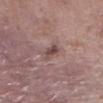workup: total-body-photography surveillance lesion; no biopsy | TBP lesion metrics: an average lesion color of about L≈46 a*≈18 b*≈19 (CIELAB), roughly 10 lightness units darker than nearby skin, and a lesion-to-skin contrast of about 7.5 (normalized; higher = more distinct) | imaging modality: 15 mm crop, total-body photography | lighting: white-light illumination | location: the left lower leg | subject: female, aged around 85 or older.